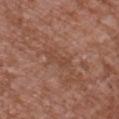Recorded during total-body skin imaging; not selected for excision or biopsy.
The lesion's longest dimension is about 3 mm.
Located on the chest.
Captured under white-light illumination.
The lesion-visualizer software estimated a border-irregularity index near 4.5/10, internal color variation of about 0.5 on a 0–10 scale, and peripheral color asymmetry of about 0.
A male subject, aged 43–47.
A close-up tile cropped from a whole-body skin photograph, about 15 mm across.